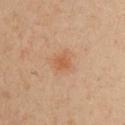biopsy_status: not biopsied; imaged during a skin examination
site: chest
image:
  source: total-body photography crop
  field_of_view_mm: 15
automated_metrics:
  area_mm2_approx: 3.0
  eccentricity: 0.4
  shape_asymmetry: 0.2
  border_irregularity_0_10: 2.0
  color_variation_0_10: 1.0
  peripheral_color_asymmetry: 0.5
lighting: cross-polarized
patient:
  sex: female
  age_approx: 40
lesion_size:
  long_diameter_mm_approx: 2.0